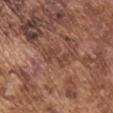Q: Was this lesion biopsied?
A: catalogued during a skin exam; not biopsied
Q: Lesion size?
A: ~4 mm (longest diameter)
Q: Who is the patient?
A: male, aged 73–77
Q: Illumination type?
A: white-light
Q: What is the imaging modality?
A: ~15 mm tile from a whole-body skin photo
Q: Where on the body is the lesion?
A: the left upper arm